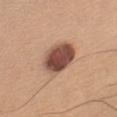biopsy status=catalogued during a skin exam; not biopsied | image=15 mm crop, total-body photography | patient=female, approximately 45 years of age | location=the left upper arm.